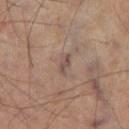biopsy status: no biopsy performed (imaged during a skin exam) | body site: the left thigh | acquisition: ~15 mm crop, total-body skin-cancer survey | patient: male, about 65 years old | illumination: cross-polarized illumination | lesion diameter: ≈3 mm | image-analysis metrics: a lesion area of about 3 mm²; about 8 CIELAB-L* units darker than the surrounding skin and a lesion-to-skin contrast of about 7 (normalized; higher = more distinct); a border-irregularity index near 3.5/10 and a color-variation rating of about 0.5/10; an automated nevus-likeness rating near 0 out of 100 and a detector confidence of about 90 out of 100 that the crop contains a lesion.